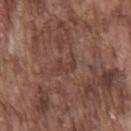biopsy_status: not biopsied; imaged during a skin examination
site: front of the torso
patient:
  sex: male
  age_approx: 75
lighting: white-light
image:
  source: total-body photography crop
  field_of_view_mm: 15